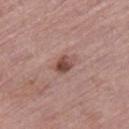The lesion was tiled from a total-body skin photograph and was not biopsied.
On the right thigh.
A roughly 15 mm field-of-view crop from a total-body skin photograph.
The lesion's longest dimension is about 2.5 mm.
A female patient, in their mid- to late 60s.
Captured under white-light illumination.